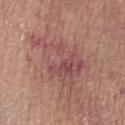Context:
An algorithmic analysis of the crop reported an outline eccentricity of about 0.8 (0 = round, 1 = elongated) and a symmetry-axis asymmetry near 0.45. The analysis additionally found an average lesion color of about L≈50 a*≈24 b*≈21 (CIELAB) and about 8 CIELAB-L* units darker than the surrounding skin. And it measured a border-irregularity index near 7/10 and a within-lesion color-variation index near 7/10. The software also gave a nevus-likeness score of about 0/100 and a lesion-detection confidence of about 95/100. A female patient in their mid-60s. Cropped from a total-body skin-imaging series; the visible field is about 15 mm. On the left forearm.